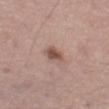Image and clinical context:
The lesion is located on the left thigh. The lesion's longest dimension is about 3 mm. A close-up tile cropped from a whole-body skin photograph, about 15 mm across. Imaged with white-light lighting. A male subject, aged approximately 75.